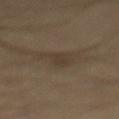{"biopsy_status": "not biopsied; imaged during a skin examination", "lesion_size": {"long_diameter_mm_approx": 3.0}, "patient": {"sex": "male", "age_approx": 60}, "image": {"source": "total-body photography crop", "field_of_view_mm": 15}, "site": "mid back"}